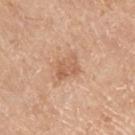{
  "biopsy_status": "not biopsied; imaged during a skin examination",
  "lighting": "white-light",
  "patient": {
    "sex": "male",
    "age_approx": 70
  },
  "automated_metrics": {
    "area_mm2_approx": 5.5,
    "eccentricity": 0.7,
    "shape_asymmetry": 0.35,
    "border_irregularity_0_10": 3.5,
    "color_variation_0_10": 2.5,
    "peripheral_color_asymmetry": 1.0
  },
  "image": {
    "source": "total-body photography crop",
    "field_of_view_mm": 15
  },
  "site": "left upper arm"
}